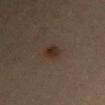biopsy status: catalogued during a skin exam; not biopsied
site: the right upper arm
acquisition: ~15 mm tile from a whole-body skin photo
patient: male, aged 33–37
lighting: cross-polarized
automated lesion analysis: an area of roughly 3.5 mm², a shape eccentricity near 0.8, and a shape-asymmetry score of about 0.2 (0 = symmetric); a border-irregularity rating of about 2/10, a color-variation rating of about 3.5/10, and radial color variation of about 1.5; a detector confidence of about 100 out of 100 that the crop contains a lesion